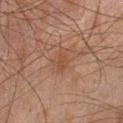A male subject about 45 years old. About 3 mm across. Imaged with cross-polarized lighting. A 15 mm close-up tile from a total-body photography series done for melanoma screening. On the left lower leg.